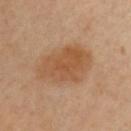follow-up: no biopsy performed (imaged during a skin exam)
location: the chest
image: total-body-photography crop, ~15 mm field of view
patient: male, aged 48 to 52
size: ≈6 mm
image-analysis metrics: a border-irregularity rating of about 2.5/10 and internal color variation of about 3 on a 0–10 scale; a nevus-likeness score of about 45/100 and lesion-presence confidence of about 100/100